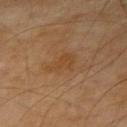Q: Was this lesion biopsied?
A: total-body-photography surveillance lesion; no biopsy
Q: What did automated image analysis measure?
A: an eccentricity of roughly 0.75 and a symmetry-axis asymmetry near 0.4; a lesion-detection confidence of about 100/100
Q: What is the anatomic site?
A: the left forearm
Q: What are the patient's age and sex?
A: male, aged approximately 70
Q: How large is the lesion?
A: ≈3.5 mm
Q: What is the imaging modality?
A: ~15 mm tile from a whole-body skin photo
Q: Illumination type?
A: cross-polarized illumination